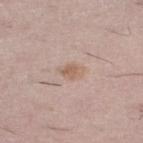Clinical impression:
No biopsy was performed on this lesion — it was imaged during a full skin examination and was not determined to be concerning.
Context:
The recorded lesion diameter is about 2.5 mm. The lesion is on the left thigh. A region of skin cropped from a whole-body photographic capture, roughly 15 mm wide. A male subject, aged around 55.